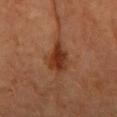<record>
<automated_metrics>
  <cielab_L>34</cielab_L>
  <cielab_a>24</cielab_a>
  <cielab_b>32</cielab_b>
  <vs_skin_darker_L>11.0</vs_skin_darker_L>
  <vs_skin_contrast_norm>9.5</vs_skin_contrast_norm>
  <color_variation_0_10>4.5</color_variation_0_10>
  <nevus_likeness_0_100>90</nevus_likeness_0_100>
  <lesion_detection_confidence_0_100>100</lesion_detection_confidence_0_100>
</automated_metrics>
<patient>
  <sex>female</sex>
  <age_approx>65</age_approx>
</patient>
<site>right forearm</site>
<image>
  <source>total-body photography crop</source>
  <field_of_view_mm>15</field_of_view_mm>
</image>
</record>